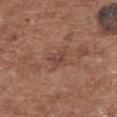{
  "biopsy_status": "not biopsied; imaged during a skin examination",
  "lighting": "white-light",
  "automated_metrics": {
    "eccentricity": 0.7,
    "cielab_L": 44,
    "cielab_a": 21,
    "cielab_b": 25,
    "vs_skin_darker_L": 7.0,
    "vs_skin_contrast_norm": 5.5,
    "lesion_detection_confidence_0_100": 100
  },
  "image": {
    "source": "total-body photography crop",
    "field_of_view_mm": 15
  },
  "lesion_size": {
    "long_diameter_mm_approx": 3.0
  },
  "site": "upper back",
  "patient": {
    "sex": "female",
    "age_approx": 75
  }
}